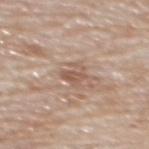Findings:
– workup — imaged on a skin check; not biopsied
– size — ≈2.5 mm
– illumination — white-light illumination
– patient — male, aged 78–82
– anatomic site — the mid back
– image — total-body-photography crop, ~15 mm field of view